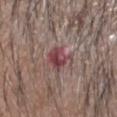Recorded during total-body skin imaging; not selected for excision or biopsy. Measured at roughly 3.5 mm in maximum diameter. Cropped from a total-body skin-imaging series; the visible field is about 15 mm. The lesion is located on the head or neck. A male subject approximately 50 years of age. Imaged with white-light lighting.